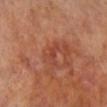Q: Is there a histopathology result?
A: catalogued during a skin exam; not biopsied
Q: What is the imaging modality?
A: 15 mm crop, total-body photography
Q: Where on the body is the lesion?
A: the left leg
Q: What are the patient's age and sex?
A: female, aged around 65